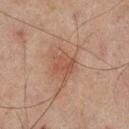Assessment:
The lesion was tiled from a total-body skin photograph and was not biopsied.
Clinical summary:
Approximately 2.5 mm at its widest. Automated tile analysis of the lesion measured an eccentricity of roughly 0.75 and a shape-asymmetry score of about 0.25 (0 = symmetric). And it measured a border-irregularity rating of about 2.5/10, internal color variation of about 2 on a 0–10 scale, and peripheral color asymmetry of about 1. Imaged with cross-polarized lighting. A 15 mm crop from a total-body photograph taken for skin-cancer surveillance. A male subject aged 63–67. From the mid back.